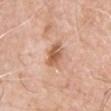No biopsy was performed on this lesion — it was imaged during a full skin examination and was not determined to be concerning. The lesion's longest dimension is about 3 mm. Captured under white-light illumination. A 15 mm close-up extracted from a 3D total-body photography capture. Located on the chest. A male patient, aged around 65.